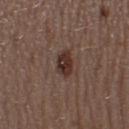workup: no biopsy performed (imaged during a skin exam) | automated metrics: a nevus-likeness score of about 95/100 and a detector confidence of about 100 out of 100 that the crop contains a lesion | image: ~15 mm crop, total-body skin-cancer survey | tile lighting: white-light illumination | lesion size: about 3 mm | location: the right thigh | patient: female, about 30 years old.